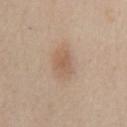Q: Was this lesion biopsied?
A: no biopsy performed (imaged during a skin exam)
Q: How was this image acquired?
A: total-body-photography crop, ~15 mm field of view
Q: Lesion size?
A: ≈2.5 mm
Q: Where on the body is the lesion?
A: the abdomen
Q: Automated lesion metrics?
A: a footprint of about 5 mm² and an eccentricity of roughly 0.55; an average lesion color of about L≈58 a*≈17 b*≈30 (CIELAB), a lesion–skin lightness drop of about 8, and a normalized lesion–skin contrast near 5.5; a border-irregularity rating of about 2.5/10 and radial color variation of about 0.5; lesion-presence confidence of about 100/100
Q: What lighting was used for the tile?
A: white-light
Q: What are the patient's age and sex?
A: male, in their 60s